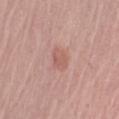Notes:
• follow-up: no biopsy performed (imaged during a skin exam)
• site: the right upper arm
• imaging modality: ~15 mm tile from a whole-body skin photo
• automated metrics: a footprint of about 5 mm², a shape eccentricity near 0.55, and a shape-asymmetry score of about 0.2 (0 = symmetric); border irregularity of about 2 on a 0–10 scale, internal color variation of about 2 on a 0–10 scale, and a peripheral color-asymmetry measure near 1
• lighting: white-light
• subject: female, aged approximately 65
• lesion size: about 2.5 mm